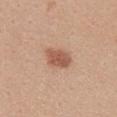Captured during whole-body skin photography for melanoma surveillance; the lesion was not biopsied.
The subject is a female in their 20s.
The total-body-photography lesion software estimated a lesion color around L≈56 a*≈22 b*≈31 in CIELAB, about 12 CIELAB-L* units darker than the surrounding skin, and a normalized border contrast of about 8. It also reported border irregularity of about 2 on a 0–10 scale. And it measured a classifier nevus-likeness of about 95/100 and a detector confidence of about 100 out of 100 that the crop contains a lesion.
From the chest.
This is a white-light tile.
The lesion's longest dimension is about 3.5 mm.
A 15 mm crop from a total-body photograph taken for skin-cancer surveillance.